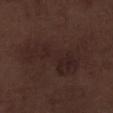A lesion tile, about 15 mm wide, cut from a 3D total-body photograph.
The patient is a male in their 70s.
Automated image analysis of the tile measured an area of roughly 25 mm², an eccentricity of roughly 0.9, and a symmetry-axis asymmetry near 0.5. And it measured peripheral color asymmetry of about 1.
Imaged with white-light lighting.
On the leg.